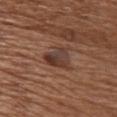Acquisition and patient details:
A female subject in their mid- to late 70s. From the front of the torso. A 15 mm crop from a total-body photograph taken for skin-cancer surveillance. The recorded lesion diameter is about 4 mm.